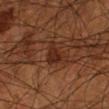Captured during whole-body skin photography for melanoma surveillance; the lesion was not biopsied.
A male subject, in their mid- to late 60s.
The lesion is on the right forearm.
This is a cross-polarized tile.
This image is a 15 mm lesion crop taken from a total-body photograph.